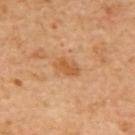Background:
This image is a 15 mm lesion crop taken from a total-body photograph. The subject is a female approximately 55 years of age. Located on the upper back. The recorded lesion diameter is about 3 mm.